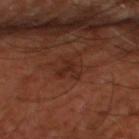Captured during whole-body skin photography for melanoma surveillance; the lesion was not biopsied.
The lesion is on the left thigh.
The subject is in their mid-60s.
Imaged with cross-polarized lighting.
The lesion's longest dimension is about 2.5 mm.
This image is a 15 mm lesion crop taken from a total-body photograph.